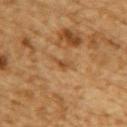Clinical impression:
This lesion was catalogued during total-body skin photography and was not selected for biopsy.
Clinical summary:
The lesion is on the upper back. The lesion-visualizer software estimated border irregularity of about 4 on a 0–10 scale, internal color variation of about 2 on a 0–10 scale, and peripheral color asymmetry of about 0.5. This image is a 15 mm lesion crop taken from a total-body photograph. Imaged with cross-polarized lighting. A male patient, aged approximately 85 or older.